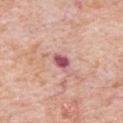Part of a total-body skin-imaging series; this lesion was reviewed on a skin check and was not flagged for biopsy. Approximately 2.5 mm at its widest. Automated tile analysis of the lesion measured peripheral color asymmetry of about 0.5. It also reported a nevus-likeness score of about 5/100 and a lesion-detection confidence of about 100/100. A male subject, in their 80s. A 15 mm close-up tile from a total-body photography series done for melanoma screening. Imaged with white-light lighting. The lesion is on the chest.